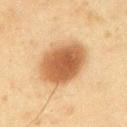Located on the right upper arm. A 15 mm close-up extracted from a 3D total-body photography capture. A male patient, approximately 45 years of age. Captured under cross-polarized illumination.